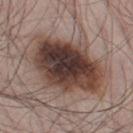notes = catalogued during a skin exam; not biopsied
illumination = white-light illumination
patient = male, aged 53 to 57
image source = ~15 mm crop, total-body skin-cancer survey
lesion diameter = about 8.5 mm
site = the left thigh